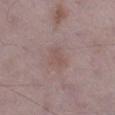Assessment: Recorded during total-body skin imaging; not selected for excision or biopsy. Clinical summary: Located on the right lower leg. Captured under white-light illumination. A male subject in their 70s. Cropped from a whole-body photographic skin survey; the tile spans about 15 mm. The lesion's longest dimension is about 2.5 mm.